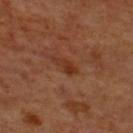Case summary:
– follow-up: total-body-photography surveillance lesion; no biopsy
– location: the upper back
– image: ~15 mm tile from a whole-body skin photo
– lighting: cross-polarized illumination
– diameter: ≈2.5 mm
– automated metrics: an average lesion color of about L≈32 a*≈23 b*≈30 (CIELAB) and a normalized lesion–skin contrast near 7; a border-irregularity rating of about 3/10, internal color variation of about 1 on a 0–10 scale, and peripheral color asymmetry of about 0.5; a classifier nevus-likeness of about 0/100 and a detector confidence of about 100 out of 100 that the crop contains a lesion
– subject: male, in their 70s